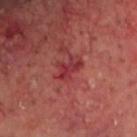No biopsy was performed on this lesion — it was imaged during a full skin examination and was not determined to be concerning. A male patient in their mid- to late 60s. The tile uses cross-polarized illumination. Cropped from a total-body skin-imaging series; the visible field is about 15 mm. Automated image analysis of the tile measured a footprint of about 4 mm², an eccentricity of roughly 0.8, and two-axis asymmetry of about 0.45. The analysis additionally found a mean CIELAB color near L≈35 a*≈35 b*≈23. And it measured a nevus-likeness score of about 0/100. The lesion is on the head or neck. Approximately 3 mm at its widest.